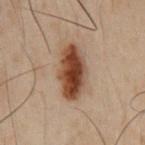The lesion was photographed on a routine skin check and not biopsied; there is no pathology result. Longest diameter approximately 6.5 mm. A male patient, in their 50s. An algorithmic analysis of the crop reported an automated nevus-likeness rating near 100 out of 100 and a detector confidence of about 100 out of 100 that the crop contains a lesion. The lesion is located on the chest. Imaged with cross-polarized lighting. Cropped from a total-body skin-imaging series; the visible field is about 15 mm.